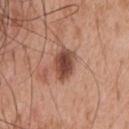follow-up: total-body-photography surveillance lesion; no biopsy
location: the chest
automated lesion analysis: a lesion color around L≈47 a*≈23 b*≈28 in CIELAB, roughly 15 lightness units darker than nearby skin, and a normalized lesion–skin contrast near 10.5; a border-irregularity rating of about 1.5/10, internal color variation of about 5 on a 0–10 scale, and radial color variation of about 1.5
subject: male, aged 53 to 57
size: ≈4 mm
image: total-body-photography crop, ~15 mm field of view
lighting: white-light illumination